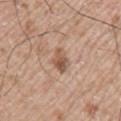Q: What are the patient's age and sex?
A: male, aged 63 to 67
Q: Illumination type?
A: white-light illumination
Q: What is the lesion's diameter?
A: ~3 mm (longest diameter)
Q: What kind of image is this?
A: ~15 mm tile from a whole-body skin photo
Q: Lesion location?
A: the arm